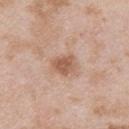<record>
  <biopsy_status>not biopsied; imaged during a skin examination</biopsy_status>
  <lesion_size>
    <long_diameter_mm_approx>3.0</long_diameter_mm_approx>
  </lesion_size>
  <lighting>white-light</lighting>
  <automated_metrics>
    <border_irregularity_0_10>2.5</border_irregularity_0_10>
    <color_variation_0_10>2.0</color_variation_0_10>
    <peripheral_color_asymmetry>0.5</peripheral_color_asymmetry>
  </automated_metrics>
  <image>
    <source>total-body photography crop</source>
    <field_of_view_mm>15</field_of_view_mm>
  </image>
  <patient>
    <sex>male</sex>
    <age_approx>25</age_approx>
  </patient>
  <site>back</site>
</record>